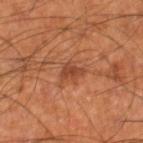Impression:
Recorded during total-body skin imaging; not selected for excision or biopsy.
Acquisition and patient details:
A male subject about 60 years old. An algorithmic analysis of the crop reported a lesion color around L≈44 a*≈26 b*≈34 in CIELAB and a lesion–skin lightness drop of about 9. And it measured lesion-presence confidence of about 100/100. The tile uses cross-polarized illumination. Cropped from a total-body skin-imaging series; the visible field is about 15 mm. Measured at roughly 2.5 mm in maximum diameter. Located on the right lower leg.